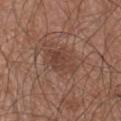Assessment: Recorded during total-body skin imaging; not selected for excision or biopsy. Context: A roughly 15 mm field-of-view crop from a total-body skin photograph. A male patient aged approximately 65. Longest diameter approximately 4 mm. This is a white-light tile. The lesion is on the chest.